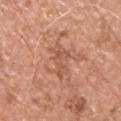Assessment:
No biopsy was performed on this lesion — it was imaged during a full skin examination and was not determined to be concerning.
Acquisition and patient details:
A region of skin cropped from a whole-body photographic capture, roughly 15 mm wide. Located on the chest. A male patient, in their mid-50s. The lesion-visualizer software estimated an area of roughly 4.5 mm², an eccentricity of roughly 0.85, and two-axis asymmetry of about 0.6. It also reported a lesion color around L≈55 a*≈24 b*≈33 in CIELAB, about 8 CIELAB-L* units darker than the surrounding skin, and a lesion-to-skin contrast of about 5.5 (normalized; higher = more distinct). It also reported a within-lesion color-variation index near 0/10 and radial color variation of about 0. The lesion's longest dimension is about 3.5 mm. The tile uses white-light illumination.